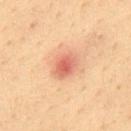Impression: No biopsy was performed on this lesion — it was imaged during a full skin examination and was not determined to be concerning. Clinical summary: The total-body-photography lesion software estimated a lesion color around L≈60 a*≈26 b*≈32 in CIELAB and a normalized lesion–skin contrast near 7. The lesion's longest dimension is about 4 mm. The lesion is on the mid back. The patient is a male approximately 35 years of age. This is a cross-polarized tile. This image is a 15 mm lesion crop taken from a total-body photograph.